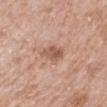The lesion was tiled from a total-body skin photograph and was not biopsied. Cropped from a whole-body photographic skin survey; the tile spans about 15 mm. An algorithmic analysis of the crop reported a mean CIELAB color near L≈56 a*≈22 b*≈29, about 10 CIELAB-L* units darker than the surrounding skin, and a normalized border contrast of about 7. The analysis additionally found border irregularity of about 2.5 on a 0–10 scale, a color-variation rating of about 4/10, and peripheral color asymmetry of about 1.5. On the right upper arm. Imaged with white-light lighting. About 3 mm across. The patient is a male roughly 70 years of age.